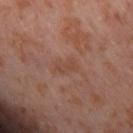Imaged during a routine full-body skin examination; the lesion was not biopsied and no histopathology is available.
This is a cross-polarized tile.
Automated tile analysis of the lesion measured border irregularity of about 3 on a 0–10 scale, a color-variation rating of about 2.5/10, and radial color variation of about 1.
The recorded lesion diameter is about 3 mm.
On the right thigh.
A region of skin cropped from a whole-body photographic capture, roughly 15 mm wide.
A female patient aged approximately 55.